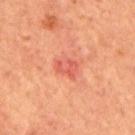Background: Imaged with cross-polarized lighting. Located on the mid back. The lesion-visualizer software estimated a footprint of about 4.5 mm², an eccentricity of roughly 0.7, and a symmetry-axis asymmetry near 0.35. A male subject, about 65 years old. A lesion tile, about 15 mm wide, cut from a 3D total-body photograph. Approximately 3 mm at its widest.